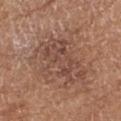Q: Was this lesion biopsied?
A: total-body-photography surveillance lesion; no biopsy
Q: How was this image acquired?
A: ~15 mm tile from a whole-body skin photo
Q: Who is the patient?
A: female, aged 73–77
Q: Where on the body is the lesion?
A: the right upper arm
Q: Illumination type?
A: white-light